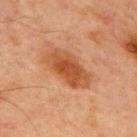biopsy status: imaged on a skin check; not biopsied.